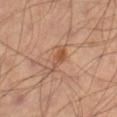Impression: Captured during whole-body skin photography for melanoma surveillance; the lesion was not biopsied. Background: This image is a 15 mm lesion crop taken from a total-body photograph. A male subject, approximately 65 years of age. Longest diameter approximately 4 mm. The lesion is on the right thigh. An algorithmic analysis of the crop reported about 8 CIELAB-L* units darker than the surrounding skin and a normalized border contrast of about 6.5. And it measured a border-irregularity rating of about 4.5/10, a color-variation rating of about 3/10, and a peripheral color-asymmetry measure near 0.5. The analysis additionally found an automated nevus-likeness rating near 20 out of 100 and a lesion-detection confidence of about 100/100. This is a cross-polarized tile.